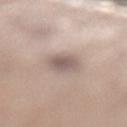Clinical impression:
Imaged during a routine full-body skin examination; the lesion was not biopsied and no histopathology is available.
Clinical summary:
A male patient in their 30s. Cropped from a total-body skin-imaging series; the visible field is about 15 mm. Located on the leg.